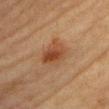Clinical summary: The tile uses cross-polarized illumination. A female patient, roughly 45 years of age. A 15 mm close-up extracted from a 3D total-body photography capture. Longest diameter approximately 4 mm. From the head or neck. The lesion-visualizer software estimated a footprint of about 8.5 mm², an eccentricity of roughly 0.7, and a shape-asymmetry score of about 0.2 (0 = symmetric). The analysis additionally found roughly 9 lightness units darker than nearby skin.